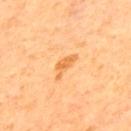| field | value |
|---|---|
| biopsy status | no biopsy performed (imaged during a skin exam) |
| patient | male, roughly 65 years of age |
| TBP lesion metrics | a lesion area of about 4 mm², a shape eccentricity near 0.9, and a symmetry-axis asymmetry near 0.5; an automated nevus-likeness rating near 5 out of 100 and a lesion-detection confidence of about 100/100 |
| imaging modality | ~15 mm tile from a whole-body skin photo |
| site | the upper back |